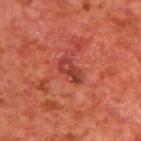Q: Is there a histopathology result?
A: imaged on a skin check; not biopsied
Q: Illumination type?
A: cross-polarized
Q: Automated lesion metrics?
A: a shape eccentricity near 0.85 and two-axis asymmetry of about 0.25; a mean CIELAB color near L≈37 a*≈31 b*≈28, roughly 8 lightness units darker than nearby skin, and a normalized lesion–skin contrast near 7; a border-irregularity rating of about 3/10, internal color variation of about 5 on a 0–10 scale, and a peripheral color-asymmetry measure near 2; an automated nevus-likeness rating near 0 out of 100 and a detector confidence of about 100 out of 100 that the crop contains a lesion
Q: Where on the body is the lesion?
A: the upper back
Q: Who is the patient?
A: male, in their mid- to late 60s
Q: How was this image acquired?
A: total-body-photography crop, ~15 mm field of view
Q: Lesion size?
A: ≈3.5 mm